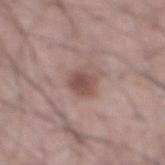Q: Was a biopsy performed?
A: catalogued during a skin exam; not biopsied
Q: What lighting was used for the tile?
A: white-light
Q: Patient demographics?
A: male, in their mid- to late 60s
Q: What did automated image analysis measure?
A: a lesion area of about 6 mm² and a shape eccentricity near 0.55; a mean CIELAB color near L≈50 a*≈19 b*≈22, about 11 CIELAB-L* units darker than the surrounding skin, and a normalized lesion–skin contrast near 7.5; a border-irregularity rating of about 2/10, internal color variation of about 2.5 on a 0–10 scale, and peripheral color asymmetry of about 0.5; a nevus-likeness score of about 55/100
Q: How large is the lesion?
A: ~3 mm (longest diameter)
Q: Where on the body is the lesion?
A: the abdomen
Q: What is the imaging modality?
A: ~15 mm crop, total-body skin-cancer survey